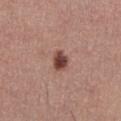A male patient aged approximately 40. A 15 mm close-up extracted from a 3D total-body photography capture. On the front of the torso. The tile uses white-light illumination. Automated tile analysis of the lesion measured an average lesion color of about L≈44 a*≈23 b*≈24 (CIELAB) and roughly 16 lightness units darker than nearby skin.